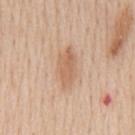<record>
<lighting>white-light</lighting>
<image>
  <source>total-body photography crop</source>
  <field_of_view_mm>15</field_of_view_mm>
</image>
<site>mid back</site>
<patient>
  <sex>male</sex>
  <age_approx>60</age_approx>
</patient>
<lesion_size>
  <long_diameter_mm_approx>5.0</long_diameter_mm_approx>
</lesion_size>
</record>